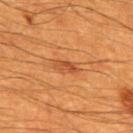No biopsy was performed on this lesion — it was imaged during a full skin examination and was not determined to be concerning.
About 3 mm across.
A 15 mm close-up tile from a total-body photography series done for melanoma screening.
Located on the mid back.
Captured under cross-polarized illumination.
A male subject, about 60 years old.
An algorithmic analysis of the crop reported an area of roughly 3.5 mm² and two-axis asymmetry of about 0.25.